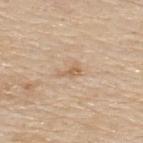Part of a total-body skin-imaging series; this lesion was reviewed on a skin check and was not flagged for biopsy.
Automated tile analysis of the lesion measured a mean CIELAB color near L≈62 a*≈17 b*≈34, about 8 CIELAB-L* units darker than the surrounding skin, and a normalized border contrast of about 6.
The lesion is located on the upper back.
Imaged with white-light lighting.
A male patient aged around 80.
A 15 mm close-up extracted from a 3D total-body photography capture.